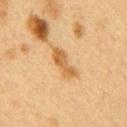Q: Was a biopsy performed?
A: total-body-photography surveillance lesion; no biopsy
Q: Lesion location?
A: the right upper arm
Q: How was the tile lit?
A: cross-polarized
Q: Automated lesion metrics?
A: a lesion area of about 6 mm², an outline eccentricity of about 0.9 (0 = round, 1 = elongated), and a shape-asymmetry score of about 0.25 (0 = symmetric); a border-irregularity index near 3/10
Q: What is the lesion's diameter?
A: about 4 mm
Q: What are the patient's age and sex?
A: female, about 40 years old
Q: What is the imaging modality?
A: ~15 mm tile from a whole-body skin photo